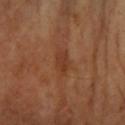biopsy_status: not biopsied; imaged during a skin examination